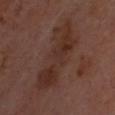notes = no biopsy performed (imaged during a skin exam) | imaging modality = ~15 mm tile from a whole-body skin photo | subject = in their mid-60s | tile lighting = cross-polarized illumination | lesion size = ≈8.5 mm | anatomic site = the head or neck.